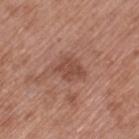Assessment: Captured during whole-body skin photography for melanoma surveillance; the lesion was not biopsied. Background: The lesion is on the left thigh. Imaged with white-light lighting. Approximately 3.5 mm at its widest. A lesion tile, about 15 mm wide, cut from a 3D total-body photograph. A female patient, roughly 75 years of age.